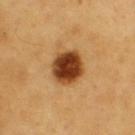Assessment:
No biopsy was performed on this lesion — it was imaged during a full skin examination and was not determined to be concerning.
Image and clinical context:
The tile uses cross-polarized illumination. Measured at roughly 4 mm in maximum diameter. On the upper back. A close-up tile cropped from a whole-body skin photograph, about 15 mm across. The patient is a male aged 58–62.